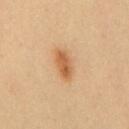biopsy_status: not biopsied; imaged during a skin examination
automated_metrics:
  border_irregularity_0_10: 2.0
  color_variation_0_10: 3.5
  peripheral_color_asymmetry: 1.0
  nevus_likeness_0_100: 100
  lesion_detection_confidence_0_100: 100
site: front of the torso
patient:
  sex: male
  age_approx: 40
lesion_size:
  long_diameter_mm_approx: 4.0
lighting: cross-polarized
image:
  source: total-body photography crop
  field_of_view_mm: 15